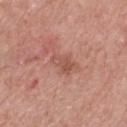Case summary:
* workup: total-body-photography surveillance lesion; no biopsy
* diameter: ≈2.5 mm
* image: 15 mm crop, total-body photography
* lighting: white-light illumination
* location: the chest
* patient: male, in their 50s